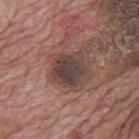Impression: The lesion was tiled from a total-body skin photograph and was not biopsied. Background: A 15 mm close-up extracted from a 3D total-body photography capture. A male subject, about 70 years old. Imaged with white-light lighting. Automated tile analysis of the lesion measured an area of roughly 12 mm², an eccentricity of roughly 0.4, and a shape-asymmetry score of about 0.2 (0 = symmetric). And it measured a mean CIELAB color near L≈40 a*≈16 b*≈19 and about 11 CIELAB-L* units darker than the surrounding skin. And it measured a border-irregularity rating of about 2/10, internal color variation of about 5 on a 0–10 scale, and radial color variation of about 1.5. About 4.5 mm across. From the back.